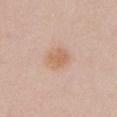Assessment:
The lesion was photographed on a routine skin check and not biopsied; there is no pathology result.
Clinical summary:
The lesion is on the chest. A female patient aged 23 to 27. Cropped from a whole-body photographic skin survey; the tile spans about 15 mm.